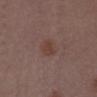- imaging modality: total-body-photography crop, ~15 mm field of view
- subject: male, aged 48–52
- anatomic site: the front of the torso